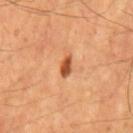• biopsy status — total-body-photography surveillance lesion; no biopsy
• body site — the mid back
• image — 15 mm crop, total-body photography
• TBP lesion metrics — a footprint of about 3 mm²; border irregularity of about 2 on a 0–10 scale, internal color variation of about 1 on a 0–10 scale, and radial color variation of about 0.5
• lighting — cross-polarized illumination
• patient — male, roughly 55 years of age
• size — about 2.5 mm